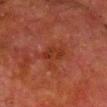Notes:
- follow-up — no biopsy performed (imaged during a skin exam)
- subject — male, aged 63 to 67
- size — ≈3 mm
- imaging modality — ~15 mm tile from a whole-body skin photo
- illumination — cross-polarized
- anatomic site — the front of the torso